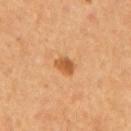– follow-up · total-body-photography surveillance lesion; no biopsy
– subject · male, aged around 55
– image · ~15 mm tile from a whole-body skin photo
– lighting · cross-polarized illumination
– body site · the mid back
– automated metrics · a lesion color around L≈52 a*≈23 b*≈39 in CIELAB, a lesion–skin lightness drop of about 10, and a normalized border contrast of about 7.5; a border-irregularity rating of about 2/10, a within-lesion color-variation index near 3/10, and peripheral color asymmetry of about 1
– lesion diameter · ≈2.5 mm